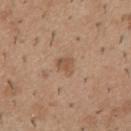Q: Was this lesion biopsied?
A: imaged on a skin check; not biopsied
Q: How was this image acquired?
A: total-body-photography crop, ~15 mm field of view
Q: Lesion size?
A: ≈2.5 mm
Q: What lighting was used for the tile?
A: white-light
Q: What did automated image analysis measure?
A: a lesion area of about 4 mm², an eccentricity of roughly 0.5, and a symmetry-axis asymmetry near 0.35; a lesion color around L≈53 a*≈18 b*≈31 in CIELAB, about 8 CIELAB-L* units darker than the surrounding skin, and a normalized lesion–skin contrast near 6; radial color variation of about 0.5
Q: Who is the patient?
A: male, aged 38 to 42
Q: Lesion location?
A: the mid back